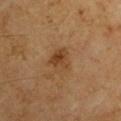workup = catalogued during a skin exam; not biopsied | size = ~3 mm (longest diameter) | image source = 15 mm crop, total-body photography | tile lighting = cross-polarized | site = the right upper arm | patient = male, about 60 years old.